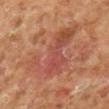Longest diameter approximately 7.5 mm.
Captured under cross-polarized illumination.
The lesion is located on the right lower leg.
Automated image analysis of the tile measured an average lesion color of about L≈46 a*≈25 b*≈29 (CIELAB), roughly 7 lightness units darker than nearby skin, and a normalized border contrast of about 5.5. It also reported a border-irregularity index near 9.5/10, internal color variation of about 5.5 on a 0–10 scale, and peripheral color asymmetry of about 1.5. The analysis additionally found a nevus-likeness score of about 0/100.
A roughly 15 mm field-of-view crop from a total-body skin photograph.
The patient is a male aged 58 to 62.